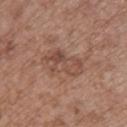No biopsy was performed on this lesion — it was imaged during a full skin examination and was not determined to be concerning.
A close-up tile cropped from a whole-body skin photograph, about 15 mm across.
This is a white-light tile.
A female patient aged 73 to 77.
The recorded lesion diameter is about 5 mm.
The lesion is located on the upper back.